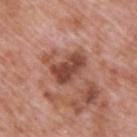{"biopsy_status": "not biopsied; imaged during a skin examination", "patient": {"sex": "male", "age_approx": 70}, "lighting": "white-light", "automated_metrics": {"area_mm2_approx": 10.0, "eccentricity": 0.8, "shape_asymmetry": 0.25, "cielab_L": 46, "cielab_a": 25, "cielab_b": 28, "vs_skin_darker_L": 13.0, "vs_skin_contrast_norm": 9.5, "border_irregularity_0_10": 3.0, "color_variation_0_10": 3.5}, "lesion_size": {"long_diameter_mm_approx": 4.5}, "site": "upper back", "image": {"source": "total-body photography crop", "field_of_view_mm": 15}}